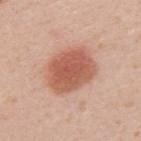Imaged during a routine full-body skin examination; the lesion was not biopsied and no histopathology is available.
A 15 mm close-up extracted from a 3D total-body photography capture.
A female patient about 30 years old.
The lesion is located on the upper back.